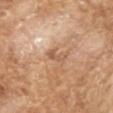Imaged during a routine full-body skin examination; the lesion was not biopsied and no histopathology is available.
A roughly 15 mm field-of-view crop from a total-body skin photograph.
Longest diameter approximately 3 mm.
Captured under cross-polarized illumination.
A male subject roughly 65 years of age.